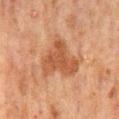| feature | finding |
|---|---|
| biopsy status | total-body-photography surveillance lesion; no biopsy |
| body site | the back |
| illumination | cross-polarized |
| automated lesion analysis | a footprint of about 18 mm², a shape eccentricity near 0.45, and a symmetry-axis asymmetry near 0.5; a lesion color around L≈41 a*≈20 b*≈29 in CIELAB, roughly 8 lightness units darker than nearby skin, and a lesion-to-skin contrast of about 7 (normalized; higher = more distinct) |
| acquisition | 15 mm crop, total-body photography |
| patient | male, about 60 years old |